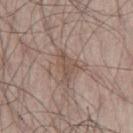Clinical impression: The lesion was tiled from a total-body skin photograph and was not biopsied. Context: The tile uses white-light illumination. A lesion tile, about 15 mm wide, cut from a 3D total-body photograph. A male patient in their mid-50s. The lesion is on the left thigh. Measured at roughly 4.5 mm in maximum diameter.